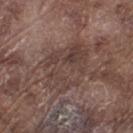Case summary:
• notes: imaged on a skin check; not biopsied
• automated metrics: an area of roughly 22 mm², a shape eccentricity near 0.75, and a shape-asymmetry score of about 0.2 (0 = symmetric); a lesion–skin lightness drop of about 7 and a normalized border contrast of about 6; border irregularity of about 3.5 on a 0–10 scale, a within-lesion color-variation index near 5/10, and radial color variation of about 2; an automated nevus-likeness rating near 0 out of 100 and a detector confidence of about 65 out of 100 that the crop contains a lesion
• site: the left thigh
• subject: male, roughly 75 years of age
• acquisition: 15 mm crop, total-body photography
• illumination: white-light illumination
• lesion size: about 7 mm